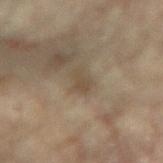Q: Is there a histopathology result?
A: catalogued during a skin exam; not biopsied
Q: What did automated image analysis measure?
A: a lesion area of about 3 mm², a shape eccentricity near 0.8, and a shape-asymmetry score of about 0.4 (0 = symmetric); a border-irregularity index near 4/10, a within-lesion color-variation index near 1/10, and a peripheral color-asymmetry measure near 0.5; an automated nevus-likeness rating near 0 out of 100 and a detector confidence of about 80 out of 100 that the crop contains a lesion
Q: What is the imaging modality?
A: total-body-photography crop, ~15 mm field of view
Q: What lighting was used for the tile?
A: cross-polarized illumination
Q: What is the lesion's diameter?
A: about 2.5 mm
Q: Lesion location?
A: the right arm
Q: Who is the patient?
A: female, about 80 years old